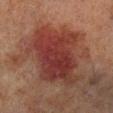Imaged during a routine full-body skin examination; the lesion was not biopsied and no histopathology is available.
The lesion is located on the leg.
An algorithmic analysis of the crop reported a border-irregularity index near 4.5/10, internal color variation of about 5.5 on a 0–10 scale, and radial color variation of about 2.
A male subject, approximately 70 years of age.
The tile uses cross-polarized illumination.
The lesion's longest dimension is about 9.5 mm.
This image is a 15 mm lesion crop taken from a total-body photograph.